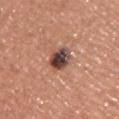Notes:
• workup · no biopsy performed (imaged during a skin exam)
• subject · male, aged 43 to 47
• anatomic site · the chest
• imaging modality · ~15 mm tile from a whole-body skin photo
• size · about 3.5 mm